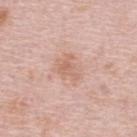Context:
On the upper back. A female subject roughly 65 years of age. The total-body-photography lesion software estimated a lesion area of about 4 mm², an outline eccentricity of about 0.65 (0 = round, 1 = elongated), and a shape-asymmetry score of about 0.55 (0 = symmetric). The analysis additionally found a lesion color around L≈63 a*≈21 b*≈29 in CIELAB, roughly 8 lightness units darker than nearby skin, and a normalized border contrast of about 5.5. The analysis additionally found a nevus-likeness score of about 0/100 and a lesion-detection confidence of about 100/100. This is a white-light tile. A region of skin cropped from a whole-body photographic capture, roughly 15 mm wide.